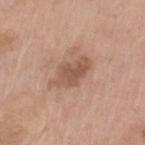The lesion was photographed on a routine skin check and not biopsied; there is no pathology result.
Automated tile analysis of the lesion measured a mean CIELAB color near L≈54 a*≈20 b*≈28 and a normalized lesion–skin contrast near 7. The software also gave internal color variation of about 3 on a 0–10 scale and radial color variation of about 1. The software also gave a classifier nevus-likeness of about 20/100.
The tile uses white-light illumination.
On the left upper arm.
A lesion tile, about 15 mm wide, cut from a 3D total-body photograph.
The lesion's longest dimension is about 4.5 mm.
A female subject, roughly 75 years of age.